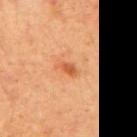This lesion was catalogued during total-body skin photography and was not selected for biopsy.
A region of skin cropped from a whole-body photographic capture, roughly 15 mm wide.
Automated image analysis of the tile measured a footprint of about 2.5 mm², an eccentricity of roughly 0.85, and a symmetry-axis asymmetry near 0.3. The analysis additionally found an average lesion color of about L≈59 a*≈31 b*≈43 (CIELAB) and a normalized border contrast of about 7.5. And it measured a classifier nevus-likeness of about 35/100 and a detector confidence of about 100 out of 100 that the crop contains a lesion.
The lesion is on the right upper arm.
The tile uses cross-polarized illumination.
A female subject, roughly 40 years of age.